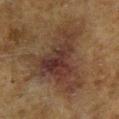This lesion was catalogued during total-body skin photography and was not selected for biopsy. The lesion is located on the right lower leg. A male patient roughly 60 years of age. Cropped from a whole-body photographic skin survey; the tile spans about 15 mm. The recorded lesion diameter is about 10.5 mm.